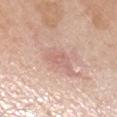- biopsy status: imaged on a skin check; not biopsied
- size: about 2 mm
- acquisition: ~15 mm tile from a whole-body skin photo
- image-analysis metrics: peripheral color asymmetry of about 0; a nevus-likeness score of about 0/100
- subject: female, in their mid-60s
- lighting: white-light
- body site: the left forearm